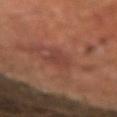Imaged during a routine full-body skin examination; the lesion was not biopsied and no histopathology is available. The tile uses cross-polarized illumination. On the left forearm. The patient is a male in their mid-60s. The lesion's longest dimension is about 3 mm. This image is a 15 mm lesion crop taken from a total-body photograph. The total-body-photography lesion software estimated a classifier nevus-likeness of about 0/100 and a detector confidence of about 95 out of 100 that the crop contains a lesion.